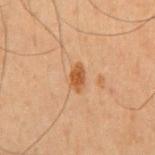| feature | finding |
|---|---|
| patient | male, aged around 50 |
| lesion diameter | ≈3 mm |
| tile lighting | cross-polarized |
| body site | the chest |
| acquisition | ~15 mm crop, total-body skin-cancer survey |